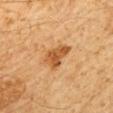Impression: Part of a total-body skin-imaging series; this lesion was reviewed on a skin check and was not flagged for biopsy. Acquisition and patient details: A male patient, in their 60s. About 4 mm across. The lesion is located on the mid back. A 15 mm crop from a total-body photograph taken for skin-cancer surveillance.